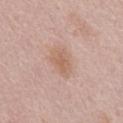Impression:
The lesion was tiled from a total-body skin photograph and was not biopsied.
Background:
A male subject aged approximately 65. A roughly 15 mm field-of-view crop from a total-body skin photograph. Approximately 3.5 mm at its widest. Located on the mid back. The tile uses white-light illumination. The total-body-photography lesion software estimated an area of roughly 6.5 mm², an eccentricity of roughly 0.75, and two-axis asymmetry of about 0.2. The software also gave a border-irregularity rating of about 2/10 and a color-variation rating of about 2.5/10. It also reported lesion-presence confidence of about 100/100.